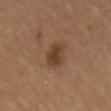The lesion was photographed on a routine skin check and not biopsied; there is no pathology result.
Approximately 4 mm at its widest.
The lesion is on the chest.
The tile uses cross-polarized illumination.
A roughly 15 mm field-of-view crop from a total-body skin photograph.